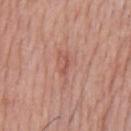A 15 mm crop from a total-body photograph taken for skin-cancer surveillance. The patient is a male about 55 years old. This is a white-light tile. The lesion is located on the chest. The recorded lesion diameter is about 2.5 mm.